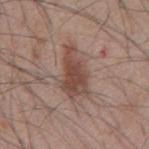{
  "biopsy_status": "not biopsied; imaged during a skin examination",
  "lighting": "white-light",
  "site": "mid back",
  "image": {
    "source": "total-body photography crop",
    "field_of_view_mm": 15
  },
  "patient": {
    "sex": "male",
    "age_approx": 45
  },
  "lesion_size": {
    "long_diameter_mm_approx": 6.0
  }
}